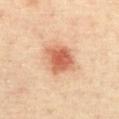<record>
<biopsy_status>not biopsied; imaged during a skin examination</biopsy_status>
<image>
  <source>total-body photography crop</source>
  <field_of_view_mm>15</field_of_view_mm>
</image>
<patient>
  <sex>female</sex>
  <age_approx>45</age_approx>
</patient>
<site>abdomen</site>
</record>